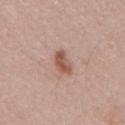Assessment: Recorded during total-body skin imaging; not selected for excision or biopsy. Acquisition and patient details: This image is a 15 mm lesion crop taken from a total-body photograph. The lesion is on the back. Longest diameter approximately 3.5 mm. A male subject in their mid-50s. Imaged with white-light lighting.